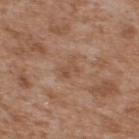The lesion was photographed on a routine skin check and not biopsied; there is no pathology result. This is a white-light tile. Measured at roughly 3 mm in maximum diameter. A male subject, about 65 years old. A region of skin cropped from a whole-body photographic capture, roughly 15 mm wide. Located on the upper back.